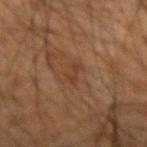Captured during whole-body skin photography for melanoma surveillance; the lesion was not biopsied.
Approximately 2.5 mm at its widest.
Imaged with cross-polarized lighting.
The lesion is located on the left forearm.
Cropped from a whole-body photographic skin survey; the tile spans about 15 mm.
A male subject, roughly 65 years of age.